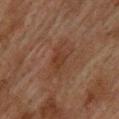Part of a total-body skin-imaging series; this lesion was reviewed on a skin check and was not flagged for biopsy. From the upper back. This is a cross-polarized tile. The lesion's longest dimension is about 2.5 mm. A male subject about 75 years old. Automated image analysis of the tile measured an outline eccentricity of about 0.65 (0 = round, 1 = elongated) and two-axis asymmetry of about 0.25. And it measured roughly 5 lightness units darker than nearby skin. And it measured an automated nevus-likeness rating near 0 out of 100 and a detector confidence of about 100 out of 100 that the crop contains a lesion. A 15 mm close-up extracted from a 3D total-body photography capture.